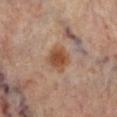biopsy_status: not biopsied; imaged during a skin examination
image:
  source: total-body photography crop
  field_of_view_mm: 15
patient:
  sex: female
  age_approx: 60
site: right lower leg